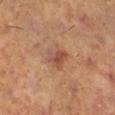Impression:
Part of a total-body skin-imaging series; this lesion was reviewed on a skin check and was not flagged for biopsy.
Acquisition and patient details:
The total-body-photography lesion software estimated an eccentricity of roughly 0.55 and a shape-asymmetry score of about 0.2 (0 = symmetric). And it measured a lesion color around L≈50 a*≈23 b*≈31 in CIELAB. The software also gave a border-irregularity rating of about 2.5/10, a color-variation rating of about 5.5/10, and peripheral color asymmetry of about 1.5. A 15 mm close-up extracted from a 3D total-body photography capture. On the right lower leg. A female subject aged 38 to 42. This is a cross-polarized tile. Approximately 3 mm at its widest.